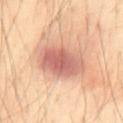Clinical impression:
The lesion was photographed on a routine skin check and not biopsied; there is no pathology result.
Clinical summary:
This image is a 15 mm lesion crop taken from a total-body photograph. The lesion's longest dimension is about 6.5 mm. From the back. Captured under cross-polarized illumination. A male patient aged approximately 55.